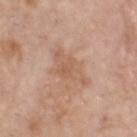Recorded during total-body skin imaging; not selected for excision or biopsy. This image is a 15 mm lesion crop taken from a total-body photograph. Captured under white-light illumination. A male subject about 70 years old. The lesion is on the head or neck. The lesion's longest dimension is about 5.5 mm.